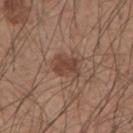{
  "biopsy_status": "not biopsied; imaged during a skin examination",
  "image": {
    "source": "total-body photography crop",
    "field_of_view_mm": 15
  },
  "automated_metrics": {
    "cielab_L": 42,
    "cielab_a": 20,
    "cielab_b": 27,
    "vs_skin_darker_L": 9.0,
    "border_irregularity_0_10": 4.0,
    "color_variation_0_10": 2.0,
    "peripheral_color_asymmetry": 0.5,
    "nevus_likeness_0_100": 35
  },
  "patient": {
    "sex": "male",
    "age_approx": 55
  },
  "lesion_size": {
    "long_diameter_mm_approx": 3.0
  },
  "site": "left forearm"
}